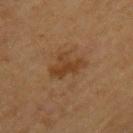notes=imaged on a skin check; not biopsied | image source=~15 mm tile from a whole-body skin photo | illumination=cross-polarized illumination | site=the upper back | patient=male, aged 58–62 | diameter=~4.5 mm (longest diameter).